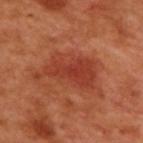workup = imaged on a skin check; not biopsied
subject = male, aged 48–52
image source = ~15 mm crop, total-body skin-cancer survey
body site = the upper back